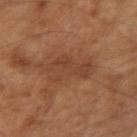Q: Is there a histopathology result?
A: imaged on a skin check; not biopsied
Q: What kind of image is this?
A: ~15 mm tile from a whole-body skin photo
Q: Patient demographics?
A: male, aged 63 to 67
Q: What lighting was used for the tile?
A: cross-polarized illumination
Q: What is the anatomic site?
A: the left arm
Q: What is the lesion's diameter?
A: ≈5 mm